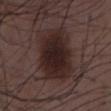Captured during whole-body skin photography for melanoma surveillance; the lesion was not biopsied.
The patient is a male aged 48 to 52.
Approximately 6.5 mm at its widest.
Captured under white-light illumination.
A lesion tile, about 15 mm wide, cut from a 3D total-body photograph.